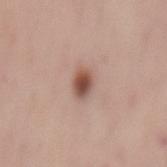The lesion was photographed on a routine skin check and not biopsied; there is no pathology result. A female patient, aged 63 to 67. About 3 mm across. Imaged with white-light lighting. Automated tile analysis of the lesion measured a border-irregularity rating of about 1.5/10 and internal color variation of about 5 on a 0–10 scale. The analysis additionally found a classifier nevus-likeness of about 100/100 and a lesion-detection confidence of about 100/100. The lesion is on the mid back. A region of skin cropped from a whole-body photographic capture, roughly 15 mm wide.